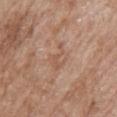Findings:
– biopsy status · catalogued during a skin exam; not biopsied
– imaging modality · ~15 mm crop, total-body skin-cancer survey
– location · the upper back
– diameter · ≈3.5 mm
– patient · female, aged approximately 85 or older